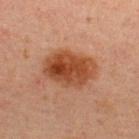Recorded during total-body skin imaging; not selected for excision or biopsy. From the upper back. This image is a 15 mm lesion crop taken from a total-body photograph. An algorithmic analysis of the crop reported an area of roughly 19 mm², an eccentricity of roughly 0.75, and a shape-asymmetry score of about 0.15 (0 = symmetric). The software also gave a lesion color around L≈38 a*≈22 b*≈30 in CIELAB and a normalized border contrast of about 10. The software also gave a within-lesion color-variation index near 6/10 and peripheral color asymmetry of about 2. A male subject, aged 28–32. Approximately 6 mm at its widest. This is a cross-polarized tile.